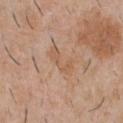{"biopsy_status": "not biopsied; imaged during a skin examination", "site": "chest", "patient": {"sex": "male", "age_approx": 30}, "automated_metrics": {"area_mm2_approx": 6.0, "eccentricity": 0.9, "shape_asymmetry": 0.4, "border_irregularity_0_10": 5.0, "nevus_likeness_0_100": 0, "lesion_detection_confidence_0_100": 100}, "image": {"source": "total-body photography crop", "field_of_view_mm": 15}}